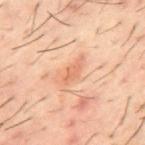notes: no biopsy performed (imaged during a skin exam); subject: male, aged 58 to 62; diameter: about 3.5 mm; tile lighting: cross-polarized illumination; image: 15 mm crop, total-body photography; location: the mid back.